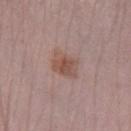A female patient aged approximately 50. Cropped from a total-body skin-imaging series; the visible field is about 15 mm. From the left lower leg. This is a white-light tile.